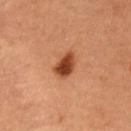Part of a total-body skin-imaging series; this lesion was reviewed on a skin check and was not flagged for biopsy.
On the abdomen.
A lesion tile, about 15 mm wide, cut from a 3D total-body photograph.
A male patient roughly 50 years of age.